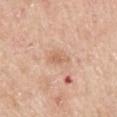notes: no biopsy performed (imaged during a skin exam) | anatomic site: the mid back | subject: male, aged approximately 60 | tile lighting: white-light | image source: ~15 mm tile from a whole-body skin photo | automated metrics: a footprint of about 3.5 mm², an outline eccentricity of about 0.85 (0 = round, 1 = elongated), and a symmetry-axis asymmetry near 0.3; a mean CIELAB color near L≈64 a*≈21 b*≈33, a lesion–skin lightness drop of about 8, and a normalized lesion–skin contrast near 5.5; a nevus-likeness score of about 0/100 and lesion-presence confidence of about 100/100 | lesion size: about 3 mm.